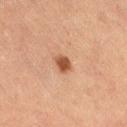Q: Is there a histopathology result?
A: catalogued during a skin exam; not biopsied
Q: What are the patient's age and sex?
A: female, aged 58–62
Q: What did automated image analysis measure?
A: a footprint of about 3.5 mm² and an eccentricity of roughly 0.8; a border-irregularity rating of about 2.5/10 and radial color variation of about 0.5; a nevus-likeness score of about 95/100
Q: What is the anatomic site?
A: the leg
Q: How large is the lesion?
A: about 2.5 mm
Q: What lighting was used for the tile?
A: cross-polarized
Q: How was this image acquired?
A: ~15 mm crop, total-body skin-cancer survey